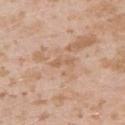The lesion was photographed on a routine skin check and not biopsied; there is no pathology result. The subject is a female aged 23 to 27. This is a white-light tile. From the left upper arm. A region of skin cropped from a whole-body photographic capture, roughly 15 mm wide. Automated image analysis of the tile measured a border-irregularity index near 4.5/10 and peripheral color asymmetry of about 0. Longest diameter approximately 3 mm.